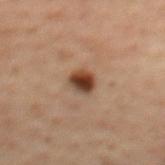This lesion was catalogued during total-body skin photography and was not selected for biopsy. A 15 mm crop from a total-body photograph taken for skin-cancer surveillance. On the mid back. Imaged with cross-polarized lighting. The patient is a male aged 53–57.